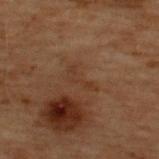Clinical impression: This lesion was catalogued during total-body skin photography and was not selected for biopsy. Clinical summary: The lesion is located on the upper back. The lesion-visualizer software estimated an area of roughly 4 mm², an outline eccentricity of about 0.9 (0 = round, 1 = elongated), and a shape-asymmetry score of about 0.6 (0 = symmetric). The analysis additionally found roughly 4 lightness units darker than nearby skin and a normalized lesion–skin contrast near 5. The software also gave a border-irregularity index near 7/10, internal color variation of about 0.5 on a 0–10 scale, and radial color variation of about 0. The subject is a male aged around 70. The recorded lesion diameter is about 3.5 mm. Captured under cross-polarized illumination. A 15 mm close-up extracted from a 3D total-body photography capture.